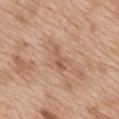  image:
    source: total-body photography crop
    field_of_view_mm: 15
  lighting: white-light
  patient:
    sex: female
    age_approx: 40
  automated_metrics:
    area_mm2_approx: 3.0
    eccentricity: 0.9
    shape_asymmetry: 0.45
    nevus_likeness_0_100: 0
    lesion_detection_confidence_0_100: 100
  site: mid back
  lesion_size:
    long_diameter_mm_approx: 3.0